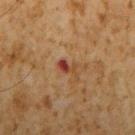Assessment: Recorded during total-body skin imaging; not selected for excision or biopsy. Context: The lesion is on the arm. A male patient, aged 58–62. A 15 mm close-up tile from a total-body photography series done for melanoma screening. The tile uses cross-polarized illumination. About 3 mm across. Automated image analysis of the tile measured roughly 9 lightness units darker than nearby skin. And it measured a border-irregularity index near 6/10, a within-lesion color-variation index near 0/10, and a peripheral color-asymmetry measure near 0.